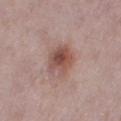{"biopsy_status": "not biopsied; imaged during a skin examination", "image": {"source": "total-body photography crop", "field_of_view_mm": 15}, "patient": {"sex": "female", "age_approx": 30}, "lesion_size": {"long_diameter_mm_approx": 4.0}, "site": "right thigh", "lighting": "white-light"}